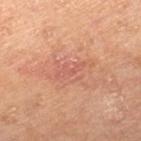biopsy status: total-body-photography surveillance lesion; no biopsy.